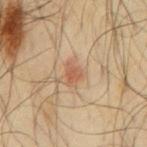Q: Was this lesion biopsied?
A: no biopsy performed (imaged during a skin exam)
Q: How was this image acquired?
A: ~15 mm crop, total-body skin-cancer survey
Q: Lesion location?
A: the mid back
Q: How was the tile lit?
A: cross-polarized
Q: Lesion size?
A: about 2.5 mm
Q: Who is the patient?
A: male, roughly 65 years of age